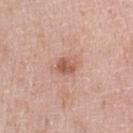Q: Is there a histopathology result?
A: imaged on a skin check; not biopsied
Q: What did automated image analysis measure?
A: a lesion-to-skin contrast of about 7.5 (normalized; higher = more distinct); a lesion-detection confidence of about 100/100
Q: Who is the patient?
A: female, aged 48–52
Q: How was the tile lit?
A: white-light illumination
Q: What is the lesion's diameter?
A: about 2.5 mm
Q: What is the imaging modality?
A: ~15 mm crop, total-body skin-cancer survey
Q: What is the anatomic site?
A: the left upper arm